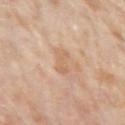{"biopsy_status": "not biopsied; imaged during a skin examination", "image": {"source": "total-body photography crop", "field_of_view_mm": 15}, "lesion_size": {"long_diameter_mm_approx": 3.0}, "patient": {"sex": "male", "age_approx": 75}, "site": "left upper arm", "lighting": "white-light", "automated_metrics": {"area_mm2_approx": 5.0, "eccentricity": 0.85, "cielab_L": 64, "cielab_a": 19, "cielab_b": 34, "vs_skin_darker_L": 7.0, "nevus_likeness_0_100": 0, "lesion_detection_confidence_0_100": 100}}